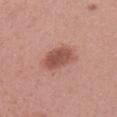<record>
<biopsy_status>not biopsied; imaged during a skin examination</biopsy_status>
<patient>
  <sex>female</sex>
  <age_approx>20</age_approx>
</patient>
<site>head or neck</site>
<lighting>white-light</lighting>
<automated_metrics>
  <cielab_L>52</cielab_L>
  <cielab_a>24</cielab_a>
  <cielab_b>27</cielab_b>
  <vs_skin_darker_L>12.0</vs_skin_darker_L>
  <vs_skin_contrast_norm>8.0</vs_skin_contrast_norm>
  <border_irregularity_0_10>1.5</border_irregularity_0_10>
  <peripheral_color_asymmetry>1.0</peripheral_color_asymmetry>
  <nevus_likeness_0_100>85</nevus_likeness_0_100>
  <lesion_detection_confidence_0_100>100</lesion_detection_confidence_0_100>
</automated_metrics>
<image>
  <source>total-body photography crop</source>
  <field_of_view_mm>15</field_of_view_mm>
</image>
<lesion_size>
  <long_diameter_mm_approx>4.5</long_diameter_mm_approx>
</lesion_size>
</record>